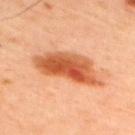| field | value |
|---|---|
| biopsy status | catalogued during a skin exam; not biopsied |
| imaging modality | total-body-photography crop, ~15 mm field of view |
| patient | male, roughly 45 years of age |
| site | the upper back |